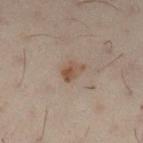Imaged during a routine full-body skin examination; the lesion was not biopsied and no histopathology is available.
Longest diameter approximately 3 mm.
A female subject, about 30 years old.
Located on the left lower leg.
A region of skin cropped from a whole-body photographic capture, roughly 15 mm wide.
Imaged with cross-polarized lighting.